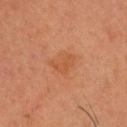Assessment: The lesion was photographed on a routine skin check and not biopsied; there is no pathology result. Background: The lesion's longest dimension is about 4 mm. This is a cross-polarized tile. From the head or neck. A 15 mm close-up tile from a total-body photography series done for melanoma screening. A male subject, in their 60s. Automated image analysis of the tile measured a lesion area of about 6.5 mm² and a shape-asymmetry score of about 0.25 (0 = symmetric). The software also gave an average lesion color of about L≈44 a*≈22 b*≈32 (CIELAB), roughly 5 lightness units darker than nearby skin, and a lesion-to-skin contrast of about 5 (normalized; higher = more distinct). And it measured a border-irregularity index near 3/10. The analysis additionally found a classifier nevus-likeness of about 5/100 and lesion-presence confidence of about 100/100.